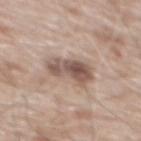Imaged during a routine full-body skin examination; the lesion was not biopsied and no histopathology is available. A male patient, aged around 80. A roughly 15 mm field-of-view crop from a total-body skin photograph. About 4.5 mm across. On the mid back. This is a white-light tile.